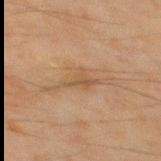No biopsy was performed on this lesion — it was imaged during a full skin examination and was not determined to be concerning.
A male patient, approximately 45 years of age.
The lesion is located on the mid back.
A 15 mm close-up extracted from a 3D total-body photography capture.